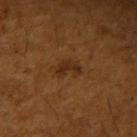Recorded during total-body skin imaging; not selected for excision or biopsy.
This is a cross-polarized tile.
Measured at roughly 3 mm in maximum diameter.
Cropped from a whole-body photographic skin survey; the tile spans about 15 mm.
A male patient, in their mid- to late 60s.
On the right upper arm.
The lesion-visualizer software estimated a lesion area of about 3.5 mm² and two-axis asymmetry of about 0.55. The analysis additionally found a border-irregularity rating of about 5.5/10, internal color variation of about 0 on a 0–10 scale, and peripheral color asymmetry of about 0. The software also gave a lesion-detection confidence of about 100/100.